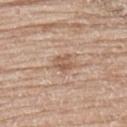Findings:
– notes — imaged on a skin check; not biopsied
– site — the upper back
– lesion size — ~2.5 mm (longest diameter)
– subject — female, in their mid- to late 80s or older
– automated lesion analysis — an area of roughly 4 mm² and a shape eccentricity near 0.6; a color-variation rating of about 3.5/10 and peripheral color asymmetry of about 1.5; a lesion-detection confidence of about 100/100
– imaging modality — ~15 mm crop, total-body skin-cancer survey
– tile lighting — white-light illumination